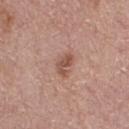biopsy status = imaged on a skin check; not biopsied
lighting = white-light
automated lesion analysis = a mean CIELAB color near L≈52 a*≈22 b*≈27, about 10 CIELAB-L* units darker than the surrounding skin, and a normalized border contrast of about 7.5; a classifier nevus-likeness of about 50/100
site = the right thigh
diameter = ≈2.5 mm
subject = female, approximately 65 years of age
imaging modality = ~15 mm crop, total-body skin-cancer survey